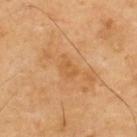Q: Was a biopsy performed?
A: imaged on a skin check; not biopsied
Q: What lighting was used for the tile?
A: cross-polarized
Q: What is the imaging modality?
A: ~15 mm tile from a whole-body skin photo
Q: Who is the patient?
A: male, approximately 70 years of age
Q: What is the lesion's diameter?
A: about 2.5 mm
Q: Where on the body is the lesion?
A: the upper back